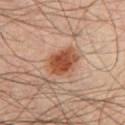  biopsy_status: not biopsied; imaged during a skin examination
  patient:
    sex: male
    age_approx: 35
  image:
    source: total-body photography crop
    field_of_view_mm: 15
  site: chest